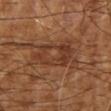Assessment:
Recorded during total-body skin imaging; not selected for excision or biopsy.
Acquisition and patient details:
Cropped from a total-body skin-imaging series; the visible field is about 15 mm. The lesion-visualizer software estimated a footprint of about 19 mm² and a shape-asymmetry score of about 0.4 (0 = symmetric). The analysis additionally found a border-irregularity rating of about 6/10 and a within-lesion color-variation index near 5.5/10. The analysis additionally found an automated nevus-likeness rating near 0 out of 100. Imaged with cross-polarized lighting. A subject aged 63–67. On the right upper arm. About 6 mm across.